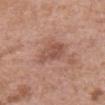This is a white-light tile. The recorded lesion diameter is about 3.5 mm. A male patient in their 70s. On the abdomen. A roughly 15 mm field-of-view crop from a total-body skin photograph.